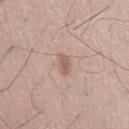No biopsy was performed on this lesion — it was imaged during a full skin examination and was not determined to be concerning.
The lesion is located on the mid back.
A male patient, aged approximately 45.
A 15 mm crop from a total-body photograph taken for skin-cancer surveillance.
The lesion's longest dimension is about 2.5 mm.
The tile uses white-light illumination.